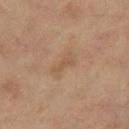Q: Is there a histopathology result?
A: catalogued during a skin exam; not biopsied
Q: What is the anatomic site?
A: the right thigh
Q: How was this image acquired?
A: ~15 mm tile from a whole-body skin photo
Q: Patient demographics?
A: female, about 55 years old